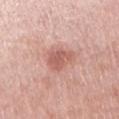<record>
  <biopsy_status>not biopsied; imaged during a skin examination</biopsy_status>
  <image>
    <source>total-body photography crop</source>
    <field_of_view_mm>15</field_of_view_mm>
  </image>
  <patient>
    <sex>female</sex>
    <age_approx>65</age_approx>
  </patient>
  <automated_metrics>
    <cielab_L>59</cielab_L>
    <cielab_a>25</cielab_a>
    <cielab_b>27</cielab_b>
    <vs_skin_contrast_norm>6.5</vs_skin_contrast_norm>
    <border_irregularity_0_10>3.0</border_irregularity_0_10>
    <peripheral_color_asymmetry>1.0</peripheral_color_asymmetry>
    <nevus_likeness_0_100>45</nevus_likeness_0_100>
    <lesion_detection_confidence_0_100>100</lesion_detection_confidence_0_100>
  </automated_metrics>
  <lesion_size>
    <long_diameter_mm_approx>3.5</long_diameter_mm_approx>
  </lesion_size>
  <site>right upper arm</site>
</record>